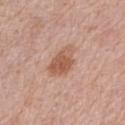Imaged during a routine full-body skin examination; the lesion was not biopsied and no histopathology is available. A region of skin cropped from a whole-body photographic capture, roughly 15 mm wide. From the right forearm. The lesion's longest dimension is about 4.5 mm. A female patient, aged 63–67.